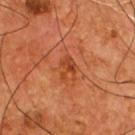This lesion was catalogued during total-body skin photography and was not selected for biopsy. A region of skin cropped from a whole-body photographic capture, roughly 15 mm wide. The lesion is located on the chest. A male subject aged approximately 55.